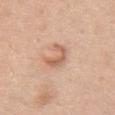follow-up — imaged on a skin check; not biopsied | automated lesion analysis — border irregularity of about 6.5 on a 0–10 scale, internal color variation of about 3.5 on a 0–10 scale, and peripheral color asymmetry of about 1; a nevus-likeness score of about 40/100 and a lesion-detection confidence of about 100/100 | image — ~15 mm tile from a whole-body skin photo | anatomic site — the chest | diameter — ≈3.5 mm | lighting — white-light illumination | patient — female, roughly 65 years of age.